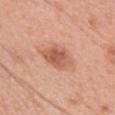<tbp_lesion>
  <image>
    <source>total-body photography crop</source>
    <field_of_view_mm>15</field_of_view_mm>
  </image>
  <patient>
    <sex>female</sex>
    <age_approx>60</age_approx>
  </patient>
  <lesion_size>
    <long_diameter_mm_approx>4.5</long_diameter_mm_approx>
  </lesion_size>
  <automated_metrics>
    <cielab_L>58</cielab_L>
    <cielab_a>25</cielab_a>
    <cielab_b>32</cielab_b>
    <vs_skin_darker_L>11.0</vs_skin_darker_L>
    <vs_skin_contrast_norm>7.5</vs_skin_contrast_norm>
    <border_irregularity_0_10>2.5</border_irregularity_0_10>
    <peripheral_color_asymmetry>1.0</peripheral_color_asymmetry>
    <lesion_detection_confidence_0_100>100</lesion_detection_confidence_0_100>
  </automated_metrics>
  <lighting>white-light</lighting>
  <site>head or neck</site>
</tbp_lesion>